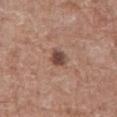follow-up=catalogued during a skin exam; not biopsied
acquisition=~15 mm crop, total-body skin-cancer survey
patient=male, aged 73 to 77
anatomic site=the abdomen